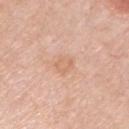Q: Was this lesion biopsied?
A: total-body-photography surveillance lesion; no biopsy
Q: What are the patient's age and sex?
A: male, approximately 55 years of age
Q: How was the tile lit?
A: white-light
Q: Lesion location?
A: the arm
Q: Automated lesion metrics?
A: a footprint of about 4.5 mm², a shape eccentricity near 0.55, and two-axis asymmetry of about 0.25; a lesion color around L≈67 a*≈21 b*≈33 in CIELAB and a lesion–skin lightness drop of about 6; a border-irregularity index near 2.5/10 and peripheral color asymmetry of about 0.5
Q: What is the imaging modality?
A: total-body-photography crop, ~15 mm field of view